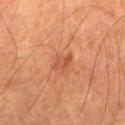The subject is a male aged approximately 65. The lesion-visualizer software estimated a symmetry-axis asymmetry near 0.35. It also reported a border-irregularity index near 3.5/10, internal color variation of about 4.5 on a 0–10 scale, and a peripheral color-asymmetry measure near 1.5. The analysis additionally found a nevus-likeness score of about 0/100 and a lesion-detection confidence of about 100/100. Cropped from a total-body skin-imaging series; the visible field is about 15 mm. The lesion is on the right thigh.